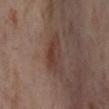Findings:
- follow-up — total-body-photography surveillance lesion; no biopsy
- subject — female, roughly 55 years of age
- size — ≈5 mm
- automated lesion analysis — an average lesion color of about L≈39 a*≈18 b*≈23 (CIELAB), roughly 7 lightness units darker than nearby skin, and a normalized lesion–skin contrast near 6.5; a border-irregularity rating of about 3/10 and a color-variation rating of about 4/10
- location — the leg
- image — ~15 mm tile from a whole-body skin photo